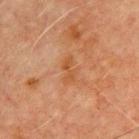follow-up: total-body-photography surveillance lesion; no biopsy
image: 15 mm crop, total-body photography
subject: male, in their 70s
illumination: cross-polarized illumination
body site: the chest